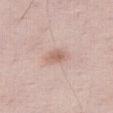No biopsy was performed on this lesion — it was imaged during a full skin examination and was not determined to be concerning.
Imaged with white-light lighting.
A close-up tile cropped from a whole-body skin photograph, about 15 mm across.
The lesion is on the leg.
Longest diameter approximately 2.5 mm.
A male subject aged 58 to 62.
The total-body-photography lesion software estimated an area of roughly 3.5 mm², a shape eccentricity near 0.75, and a shape-asymmetry score of about 0.35 (0 = symmetric). The analysis additionally found a lesion color around L≈62 a*≈20 b*≈26 in CIELAB, about 10 CIELAB-L* units darker than the surrounding skin, and a lesion-to-skin contrast of about 6.5 (normalized; higher = more distinct). It also reported an automated nevus-likeness rating near 60 out of 100 and lesion-presence confidence of about 100/100.